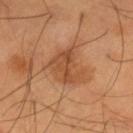Part of a total-body skin-imaging series; this lesion was reviewed on a skin check and was not flagged for biopsy. A 15 mm crop from a total-body photograph taken for skin-cancer surveillance. The patient is a male in their mid- to late 50s. Measured at roughly 4.5 mm in maximum diameter. This is a cross-polarized tile. On the left thigh.